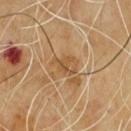This lesion was catalogued during total-body skin photography and was not selected for biopsy.
A lesion tile, about 15 mm wide, cut from a 3D total-body photograph.
Longest diameter approximately 3.5 mm.
Captured under cross-polarized illumination.
The subject is a male in their mid-60s.
The lesion is located on the front of the torso.